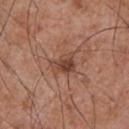Recorded during total-body skin imaging; not selected for excision or biopsy.
Cropped from a total-body skin-imaging series; the visible field is about 15 mm.
A male subject approximately 55 years of age.
The lesion is located on the chest.